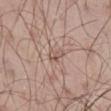Imaged during a routine full-body skin examination; the lesion was not biopsied and no histopathology is available. The tile uses white-light illumination. Approximately 2.5 mm at its widest. This image is a 15 mm lesion crop taken from a total-body photograph. An algorithmic analysis of the crop reported a border-irregularity rating of about 3/10, a within-lesion color-variation index near 1.5/10, and a peripheral color-asymmetry measure near 0.5. And it measured a nevus-likeness score of about 0/100 and a lesion-detection confidence of about 70/100. The lesion is on the right thigh. The patient is a male aged around 55.